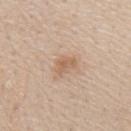This lesion was catalogued during total-body skin photography and was not selected for biopsy. The lesion is located on the mid back. The total-body-photography lesion software estimated a lesion color around L≈61 a*≈18 b*≈32 in CIELAB, a lesion–skin lightness drop of about 8, and a normalized lesion–skin contrast near 6. The software also gave a lesion-detection confidence of about 100/100. The tile uses white-light illumination. The recorded lesion diameter is about 2.5 mm. A close-up tile cropped from a whole-body skin photograph, about 15 mm across. A male patient, in their 60s.